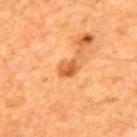| key | value |
|---|---|
| notes | no biopsy performed (imaged during a skin exam) |
| image | ~15 mm tile from a whole-body skin photo |
| automated lesion analysis | a within-lesion color-variation index near 4.5/10; a classifier nevus-likeness of about 70/100 |
| tile lighting | cross-polarized illumination |
| location | the upper back |
| subject | male, aged approximately 65 |
| lesion diameter | ≈2.5 mm |